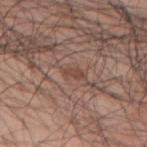Longest diameter approximately 3 mm. A lesion tile, about 15 mm wide, cut from a 3D total-body photograph. Automated tile analysis of the lesion measured a lesion color around L≈46 a*≈19 b*≈25 in CIELAB, about 7 CIELAB-L* units darker than the surrounding skin, and a lesion-to-skin contrast of about 5.5 (normalized; higher = more distinct). The software also gave border irregularity of about 3 on a 0–10 scale, a within-lesion color-variation index near 1/10, and peripheral color asymmetry of about 0. The analysis additionally found an automated nevus-likeness rating near 0 out of 100 and a lesion-detection confidence of about 55/100. On the upper back. The subject is a male aged 68 to 72.